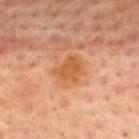biopsy status — no biopsy performed (imaged during a skin exam); subject — male, approximately 60 years of age; anatomic site — the upper back; acquisition — total-body-photography crop, ~15 mm field of view; tile lighting — cross-polarized.